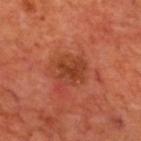This lesion was catalogued during total-body skin photography and was not selected for biopsy.
The lesion's longest dimension is about 3.5 mm.
The subject is a male aged approximately 70.
From the upper back.
Automated tile analysis of the lesion measured a footprint of about 9 mm² and an eccentricity of roughly 0.35. And it measured a border-irregularity rating of about 3.5/10, internal color variation of about 4 on a 0–10 scale, and radial color variation of about 1.5.
Imaged with cross-polarized lighting.
Cropped from a total-body skin-imaging series; the visible field is about 15 mm.